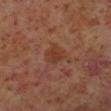Findings:
- workup: no biopsy performed (imaged during a skin exam)